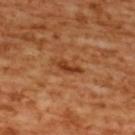Q: Was a biopsy performed?
A: total-body-photography surveillance lesion; no biopsy
Q: How was this image acquired?
A: 15 mm crop, total-body photography
Q: Patient demographics?
A: female, in their mid- to late 50s
Q: Lesion size?
A: ~3.5 mm (longest diameter)
Q: Automated lesion metrics?
A: an average lesion color of about L≈42 a*≈28 b*≈39 (CIELAB), about 10 CIELAB-L* units darker than the surrounding skin, and a normalized lesion–skin contrast near 8.5; a border-irregularity rating of about 5.5/10, internal color variation of about 1 on a 0–10 scale, and radial color variation of about 0.5
Q: Where on the body is the lesion?
A: the back